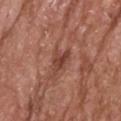| key | value |
|---|---|
| notes | no biopsy performed (imaged during a skin exam) |
| body site | the head or neck |
| lesion diameter | ~3 mm (longest diameter) |
| patient | male, in their mid-60s |
| image source | 15 mm crop, total-body photography |
| illumination | white-light |
| TBP lesion metrics | a border-irregularity index near 4.5/10, a within-lesion color-variation index near 3.5/10, and a peripheral color-asymmetry measure near 1; an automated nevus-likeness rating near 0 out of 100 and a lesion-detection confidence of about 95/100 |